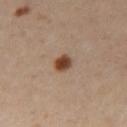This lesion was catalogued during total-body skin photography and was not selected for biopsy. A female subject about 40 years old. The tile uses cross-polarized illumination. A region of skin cropped from a whole-body photographic capture, roughly 15 mm wide. The lesion is located on the leg. About 2.5 mm across. The lesion-visualizer software estimated a footprint of about 4 mm² and a shape-asymmetry score of about 0.25 (0 = symmetric). And it measured a lesion-to-skin contrast of about 11.5 (normalized; higher = more distinct). It also reported border irregularity of about 2 on a 0–10 scale and a peripheral color-asymmetry measure near 1.5. It also reported an automated nevus-likeness rating near 100 out of 100 and a lesion-detection confidence of about 100/100.